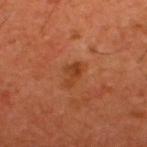follow-up=total-body-photography surveillance lesion; no biopsy | location=the upper back | TBP lesion metrics=a mean CIELAB color near L≈38 a*≈26 b*≈36, a lesion–skin lightness drop of about 7, and a normalized lesion–skin contrast near 6.5 | imaging modality=total-body-photography crop, ~15 mm field of view | patient=male, aged 58–62 | tile lighting=cross-polarized illumination | lesion diameter=~2.5 mm (longest diameter).